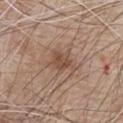Clinical summary: Longest diameter approximately 3.5 mm. On the front of the torso. A 15 mm close-up tile from a total-body photography series done for melanoma screening. A male patient approximately 80 years of age.